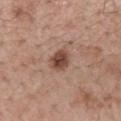Imaged during a routine full-body skin examination; the lesion was not biopsied and no histopathology is available. Cropped from a total-body skin-imaging series; the visible field is about 15 mm. A male patient aged around 55. This is a white-light tile. Automated image analysis of the tile measured a lesion area of about 6.5 mm², a shape eccentricity near 0.6, and a shape-asymmetry score of about 0.2 (0 = symmetric). The analysis additionally found a lesion color around L≈47 a*≈20 b*≈27 in CIELAB and about 13 CIELAB-L* units darker than the surrounding skin. And it measured a classifier nevus-likeness of about 95/100 and lesion-presence confidence of about 100/100. From the mid back. Longest diameter approximately 3 mm.